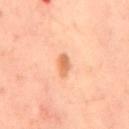notes — catalogued during a skin exam; not biopsied | site — the back | automated metrics — a shape eccentricity near 0.9 and a shape-asymmetry score of about 0.25 (0 = symmetric); a lesion color around L≈54 a*≈22 b*≈33 in CIELAB and a normalized lesion–skin contrast near 7.5; a detector confidence of about 100 out of 100 that the crop contains a lesion | acquisition — total-body-photography crop, ~15 mm field of view | size — ~2.5 mm (longest diameter) | patient — male, aged 38 to 42.